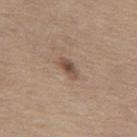Part of a total-body skin-imaging series; this lesion was reviewed on a skin check and was not flagged for biopsy.
A male patient, in their mid- to late 60s.
Longest diameter approximately 3 mm.
The lesion-visualizer software estimated an area of roughly 3.5 mm², an eccentricity of roughly 0.9, and two-axis asymmetry of about 0.3.
The lesion is located on the leg.
A 15 mm close-up extracted from a 3D total-body photography capture.